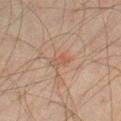On the right thigh. A male subject, in their mid- to late 40s. Cropped from a total-body skin-imaging series; the visible field is about 15 mm.